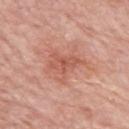workup: imaged on a skin check; not biopsied
lighting: white-light illumination
subject: female, in their mid-60s
location: the left upper arm
imaging modality: ~15 mm crop, total-body skin-cancer survey
image-analysis metrics: a border-irregularity rating of about 6.5/10 and peripheral color asymmetry of about 1